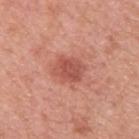No biopsy was performed on this lesion — it was imaged during a full skin examination and was not determined to be concerning.
A male patient roughly 50 years of age.
On the back.
The tile uses white-light illumination.
A close-up tile cropped from a whole-body skin photograph, about 15 mm across.